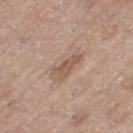Notes:
– biopsy status: catalogued during a skin exam; not biopsied
– subject: female, approximately 65 years of age
– acquisition: ~15 mm tile from a whole-body skin photo
– site: the left thigh
– lighting: white-light
– automated metrics: a lesion color around L≈55 a*≈18 b*≈28 in CIELAB, roughly 9 lightness units darker than nearby skin, and a lesion-to-skin contrast of about 6.5 (normalized; higher = more distinct); a border-irregularity rating of about 4/10, a within-lesion color-variation index near 1/10, and peripheral color asymmetry of about 0.5
– lesion size: about 3.5 mm